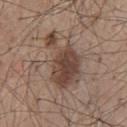biopsy_status: not biopsied; imaged during a skin examination
patient:
  sex: male
  age_approx: 55
image:
  source: total-body photography crop
  field_of_view_mm: 15
site: mid back
automated_metrics:
  area_mm2_approx: 19.0
  eccentricity: 0.85
  shape_asymmetry: 0.55
  peripheral_color_asymmetry: 1.0
  nevus_likeness_0_100: 70
  lesion_detection_confidence_0_100: 100